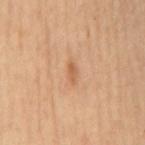Q: Was this lesion biopsied?
A: catalogued during a skin exam; not biopsied
Q: What lighting was used for the tile?
A: cross-polarized
Q: What is the lesion's diameter?
A: about 2.5 mm
Q: Patient demographics?
A: male, in their 60s
Q: What kind of image is this?
A: ~15 mm tile from a whole-body skin photo
Q: Where on the body is the lesion?
A: the mid back